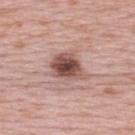lesion size = ≈4 mm; location = the upper back; patient = female, roughly 65 years of age; image source = ~15 mm crop, total-body skin-cancer survey.